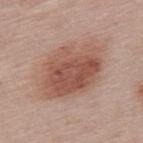The lesion was tiled from a total-body skin photograph and was not biopsied. A female patient, in their 50s. On the upper back. Measured at roughly 8 mm in maximum diameter. A close-up tile cropped from a whole-body skin photograph, about 15 mm across. The tile uses white-light illumination.